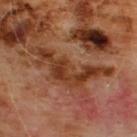Imaged during a routine full-body skin examination; the lesion was not biopsied and no histopathology is available. A 15 mm crop from a total-body photograph taken for skin-cancer surveillance. The patient is a male aged around 60. The total-body-photography lesion software estimated an automated nevus-likeness rating near 0 out of 100 and lesion-presence confidence of about 0/100. Measured at roughly 9 mm in maximum diameter. Imaged with cross-polarized lighting. The lesion is on the chest.